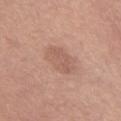notes — total-body-photography surveillance lesion; no biopsy | lesion size — ≈4 mm | patient — female, in their mid- to late 60s | acquisition — 15 mm crop, total-body photography | lighting — white-light illumination | site — the left leg.